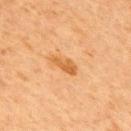  biopsy_status: not biopsied; imaged during a skin examination
  lesion_size:
    long_diameter_mm_approx: 3.5
  image:
    source: total-body photography crop
    field_of_view_mm: 15
  patient:
    sex: male
    age_approx: 65
  site: upper back
  lighting: cross-polarized